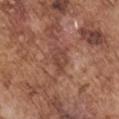Case summary:
- follow-up — catalogued during a skin exam; not biopsied
- subject — male, in their mid-70s
- automated lesion analysis — an area of roughly 6 mm², a shape eccentricity near 0.85, and a symmetry-axis asymmetry near 0.3; a classifier nevus-likeness of about 0/100 and a lesion-detection confidence of about 85/100
- acquisition — ~15 mm crop, total-body skin-cancer survey
- lesion size — ~3.5 mm (longest diameter)
- site — the left upper arm
- tile lighting — white-light illumination